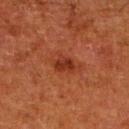The lesion was tiled from a total-body skin photograph and was not biopsied.
A 15 mm close-up tile from a total-body photography series done for melanoma screening.
Automated tile analysis of the lesion measured a footprint of about 4 mm², a shape eccentricity near 0.6, and two-axis asymmetry of about 0.25. The software also gave a lesion color around L≈27 a*≈25 b*≈28 in CIELAB, roughly 7 lightness units darker than nearby skin, and a normalized lesion–skin contrast near 8. The analysis additionally found internal color variation of about 2 on a 0–10 scale.
A male patient roughly 80 years of age.
From the right lower leg.
Measured at roughly 2.5 mm in maximum diameter.
The tile uses cross-polarized illumination.